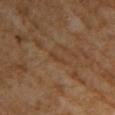No biopsy was performed on this lesion — it was imaged during a full skin examination and was not determined to be concerning. A 15 mm crop from a total-body photograph taken for skin-cancer surveillance. Measured at roughly 2.5 mm in maximum diameter. A female subject in their 70s. The lesion is on the upper back.